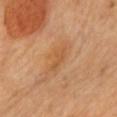Assessment:
The lesion was tiled from a total-body skin photograph and was not biopsied.
Clinical summary:
The lesion is on the chest. This is a cross-polarized tile. The lesion's longest dimension is about 3.5 mm. Cropped from a whole-body photographic skin survey; the tile spans about 15 mm. A female subject, roughly 60 years of age.